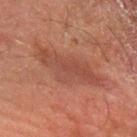Assessment: Imaged during a routine full-body skin examination; the lesion was not biopsied and no histopathology is available. Acquisition and patient details: Cropped from a whole-body photographic skin survey; the tile spans about 15 mm. The subject is a male aged 68 to 72. The recorded lesion diameter is about 8.5 mm. The lesion is on the arm.